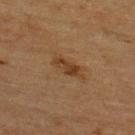workup = imaged on a skin check; not biopsied | image = ~15 mm crop, total-body skin-cancer survey | patient = male, aged 63 to 67 | illumination = cross-polarized illumination | automated metrics = a classifier nevus-likeness of about 55/100 and a lesion-detection confidence of about 100/100 | lesion diameter = ≈3.5 mm | body site = the upper back.